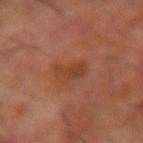Impression: Part of a total-body skin-imaging series; this lesion was reviewed on a skin check and was not flagged for biopsy. Acquisition and patient details: A male patient about 70 years old. Measured at roughly 3.5 mm in maximum diameter. Cropped from a total-body skin-imaging series; the visible field is about 15 mm. Captured under cross-polarized illumination. The lesion is on the right forearm. An algorithmic analysis of the crop reported an outline eccentricity of about 0.85 (0 = round, 1 = elongated). The software also gave a mean CIELAB color near L≈41 a*≈25 b*≈33, about 7 CIELAB-L* units darker than the surrounding skin, and a normalized lesion–skin contrast near 6.5. And it measured a nevus-likeness score of about 5/100 and a lesion-detection confidence of about 100/100.